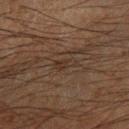Assessment: The lesion was photographed on a routine skin check and not biopsied; there is no pathology result. Context: A male subject about 65 years old. Located on the left forearm. The tile uses cross-polarized illumination. A region of skin cropped from a whole-body photographic capture, roughly 15 mm wide.